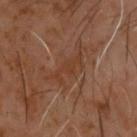Q: Is there a histopathology result?
A: no biopsy performed (imaged during a skin exam)
Q: Patient demographics?
A: male, roughly 60 years of age
Q: Lesion location?
A: the upper back
Q: What is the imaging modality?
A: 15 mm crop, total-body photography